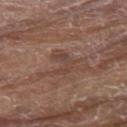workup — imaged on a skin check; not biopsied | image — total-body-photography crop, ~15 mm field of view | site — the back | subject — male, aged around 80.